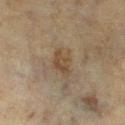{
  "biopsy_status": "not biopsied; imaged during a skin examination",
  "lesion_size": {
    "long_diameter_mm_approx": 3.0
  },
  "patient": {
    "sex": "female",
    "age_approx": 55
  },
  "lighting": "cross-polarized",
  "automated_metrics": {
    "eccentricity": 0.65,
    "border_irregularity_0_10": 2.5,
    "color_variation_0_10": 3.0,
    "peripheral_color_asymmetry": 1.0,
    "nevus_likeness_0_100": 15,
    "lesion_detection_confidence_0_100": 100
  },
  "image": {
    "source": "total-body photography crop",
    "field_of_view_mm": 15
  },
  "site": "left lower leg"
}